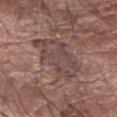notes: total-body-photography surveillance lesion; no biopsy | lighting: white-light | lesion diameter: about 6.5 mm | image: ~15 mm tile from a whole-body skin photo | location: the arm | subject: male, aged 68–72.